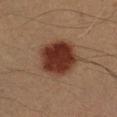Part of a total-body skin-imaging series; this lesion was reviewed on a skin check and was not flagged for biopsy.
Cropped from a total-body skin-imaging series; the visible field is about 15 mm.
From the left forearm.
A male subject, aged approximately 40.
Longest diameter approximately 5 mm.
Imaged with cross-polarized lighting.